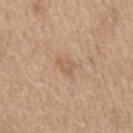Q: Lesion location?
A: the chest
Q: Who is the patient?
A: male, aged 68 to 72
Q: What kind of image is this?
A: total-body-photography crop, ~15 mm field of view